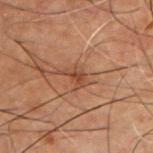Q: Was a biopsy performed?
A: imaged on a skin check; not biopsied
Q: How large is the lesion?
A: ≈2.5 mm
Q: Where on the body is the lesion?
A: the chest
Q: Automated lesion metrics?
A: two-axis asymmetry of about 0.45; an average lesion color of about L≈34 a*≈19 b*≈26 (CIELAB), roughly 7 lightness units darker than nearby skin, and a normalized lesion–skin contrast near 6.5; a classifier nevus-likeness of about 5/100 and a detector confidence of about 100 out of 100 that the crop contains a lesion
Q: Patient demographics?
A: male, aged approximately 50
Q: How was this image acquired?
A: total-body-photography crop, ~15 mm field of view